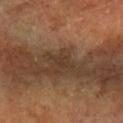No biopsy was performed on this lesion — it was imaged during a full skin examination and was not determined to be concerning.
The total-body-photography lesion software estimated an average lesion color of about L≈32 a*≈14 b*≈25 (CIELAB).
This is a cross-polarized tile.
A female subject in their 60s.
A lesion tile, about 15 mm wide, cut from a 3D total-body photograph.
The lesion is located on the right forearm.